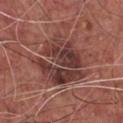Acquisition and patient details: A male patient aged around 75. Imaged with white-light lighting. Located on the chest. Automated tile analysis of the lesion measured a lesion area of about 25 mm², an eccentricity of roughly 0.4, and a symmetry-axis asymmetry near 0.3. The software also gave a classifier nevus-likeness of about 0/100 and a detector confidence of about 90 out of 100 that the crop contains a lesion. The lesion's longest dimension is about 6.5 mm. A close-up tile cropped from a whole-body skin photograph, about 15 mm across. Conclusion: Biopsy histopathology demonstrated an invasive melanoma, superficial spreading type (Breslow thickness 0.4 mm, mitotic rate <1/mm²).